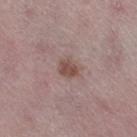Recorded during total-body skin imaging; not selected for excision or biopsy. A region of skin cropped from a whole-body photographic capture, roughly 15 mm wide. A female subject approximately 45 years of age. On the right thigh. The lesion's longest dimension is about 2.5 mm. Captured under white-light illumination. The total-body-photography lesion software estimated an outline eccentricity of about 0.45 (0 = round, 1 = elongated). The software also gave border irregularity of about 2 on a 0–10 scale, internal color variation of about 2 on a 0–10 scale, and a peripheral color-asymmetry measure near 0.5. The analysis additionally found a nevus-likeness score of about 65/100.